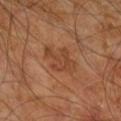Clinical impression: This lesion was catalogued during total-body skin photography and was not selected for biopsy. Background: About 5 mm across. The patient is a male roughly 60 years of age. Imaged with cross-polarized lighting. A lesion tile, about 15 mm wide, cut from a 3D total-body photograph. The lesion is located on the leg. Automated image analysis of the tile measured a mean CIELAB color near L≈41 a*≈22 b*≈32, a lesion–skin lightness drop of about 7, and a lesion-to-skin contrast of about 6 (normalized; higher = more distinct). And it measured internal color variation of about 2.5 on a 0–10 scale and peripheral color asymmetry of about 0.5. It also reported a classifier nevus-likeness of about 0/100 and a detector confidence of about 100 out of 100 that the crop contains a lesion.